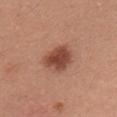Recorded during total-body skin imaging; not selected for excision or biopsy. Cropped from a whole-body photographic skin survey; the tile spans about 15 mm. The lesion is located on the front of the torso. The patient is a male aged 28–32. Longest diameter approximately 4 mm.